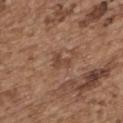Captured during whole-body skin photography for melanoma surveillance; the lesion was not biopsied.
The lesion is on the upper back.
The patient is a female roughly 65 years of age.
This image is a 15 mm lesion crop taken from a total-body photograph.
Automated image analysis of the tile measured border irregularity of about 5 on a 0–10 scale, a color-variation rating of about 1/10, and peripheral color asymmetry of about 0.5.
Measured at roughly 3 mm in maximum diameter.